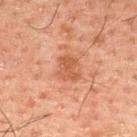notes=no biopsy performed (imaged during a skin exam)
location=the upper back
tile lighting=cross-polarized illumination
subject=male, aged around 50
image source=~15 mm crop, total-body skin-cancer survey